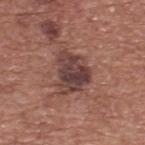The lesion was photographed on a routine skin check and not biopsied; there is no pathology result. Cropped from a whole-body photographic skin survey; the tile spans about 15 mm. The lesion is on the back. The patient is a male in their mid-70s.